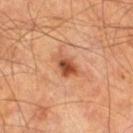From the right thigh.
A male subject, aged approximately 65.
Cropped from a whole-body photographic skin survey; the tile spans about 15 mm.